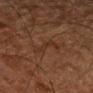Notes:
* biopsy status · total-body-photography surveillance lesion; no biopsy
* body site · the leg
* diameter · about 4.5 mm
* patient · male, aged 78–82
* illumination · cross-polarized
* image-analysis metrics · an area of roughly 5 mm² and a shape-asymmetry score of about 0.55 (0 = symmetric); an average lesion color of about L≈24 a*≈15 b*≈23 (CIELAB), roughly 4 lightness units darker than nearby skin, and a normalized border contrast of about 5; a border-irregularity rating of about 7.5/10, a within-lesion color-variation index near 1/10, and a peripheral color-asymmetry measure near 0.5; a detector confidence of about 90 out of 100 that the crop contains a lesion
* imaging modality · ~15 mm crop, total-body skin-cancer survey